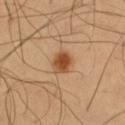This lesion was catalogued during total-body skin photography and was not selected for biopsy. A male patient aged around 55. On the leg. Cropped from a total-body skin-imaging series; the visible field is about 15 mm.